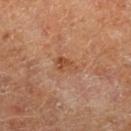Clinical impression:
The lesion was photographed on a routine skin check and not biopsied; there is no pathology result.
Clinical summary:
A roughly 15 mm field-of-view crop from a total-body skin photograph. A female patient. An algorithmic analysis of the crop reported internal color variation of about 3.5 on a 0–10 scale. The software also gave a classifier nevus-likeness of about 0/100 and a detector confidence of about 100 out of 100 that the crop contains a lesion. Located on the leg. This is a cross-polarized tile. About 3 mm across.